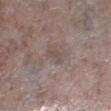notes: no biopsy performed (imaged during a skin exam) | image source: ~15 mm crop, total-body skin-cancer survey | subject: female, in their mid-80s or older | lesion size: ~2.5 mm (longest diameter) | TBP lesion metrics: a border-irregularity index near 3/10, a within-lesion color-variation index near 0.5/10, and radial color variation of about 0.5; a classifier nevus-likeness of about 0/100 and a lesion-detection confidence of about 95/100 | tile lighting: white-light | body site: the leg.